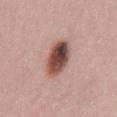Assessment:
Imaged during a routine full-body skin examination; the lesion was not biopsied and no histopathology is available.
Acquisition and patient details:
The lesion-visualizer software estimated an eccentricity of roughly 0.8. And it measured a border-irregularity rating of about 1/10, internal color variation of about 10 on a 0–10 scale, and peripheral color asymmetry of about 3.5. And it measured an automated nevus-likeness rating near 95 out of 100 and a detector confidence of about 100 out of 100 that the crop contains a lesion. A 15 mm crop from a total-body photograph taken for skin-cancer surveillance. From the back. Measured at roughly 5 mm in maximum diameter. The patient is a male approximately 45 years of age. Imaged with white-light lighting.